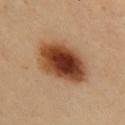Clinical impression:
Imaged during a routine full-body skin examination; the lesion was not biopsied and no histopathology is available.
Clinical summary:
Imaged with cross-polarized lighting. The total-body-photography lesion software estimated a footprint of about 24 mm², an outline eccentricity of about 0.8 (0 = round, 1 = elongated), and a shape-asymmetry score of about 0.15 (0 = symmetric). Longest diameter approximately 7 mm. A 15 mm close-up tile from a total-body photography series done for melanoma screening. Located on the chest. A female patient aged approximately 50.